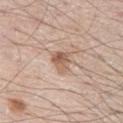The lesion was tiled from a total-body skin photograph and was not biopsied. A 15 mm crop from a total-body photograph taken for skin-cancer surveillance. A male patient, aged approximately 60. The lesion is on the left thigh.